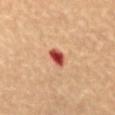This lesion was catalogued during total-body skin photography and was not selected for biopsy. Imaged with cross-polarized lighting. The subject is a female aged 68 to 72. Located on the abdomen. Longest diameter approximately 3 mm. Cropped from a total-body skin-imaging series; the visible field is about 15 mm.